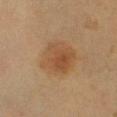{
  "biopsy_status": "not biopsied; imaged during a skin examination",
  "patient": {
    "sex": "male",
    "age_approx": 65
  },
  "site": "right lower leg",
  "image": {
    "source": "total-body photography crop",
    "field_of_view_mm": 15
  },
  "lesion_size": {
    "long_diameter_mm_approx": 4.5
  },
  "lighting": "cross-polarized"
}